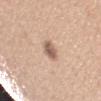• workup · imaged on a skin check; not biopsied
• lesion size · ~2.5 mm (longest diameter)
• subject · female, aged 28–32
• illumination · white-light illumination
• image · ~15 mm tile from a whole-body skin photo
• site · the left upper arm